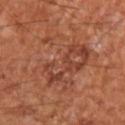Recorded during total-body skin imaging; not selected for excision or biopsy.
A patient approximately 65 years of age.
Longest diameter approximately 11 mm.
On the left upper arm.
The lesion-visualizer software estimated a lesion color around L≈45 a*≈25 b*≈31 in CIELAB.
Imaged with cross-polarized lighting.
A region of skin cropped from a whole-body photographic capture, roughly 15 mm wide.